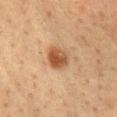Clinical impression:
No biopsy was performed on this lesion — it was imaged during a full skin examination and was not determined to be concerning.
Context:
On the chest. Automated tile analysis of the lesion measured a lesion color around L≈43 a*≈18 b*≈30 in CIELAB and a lesion-to-skin contrast of about 9 (normalized; higher = more distinct). And it measured a border-irregularity rating of about 1.5/10, a color-variation rating of about 4.5/10, and radial color variation of about 1.5. The analysis additionally found a nevus-likeness score of about 95/100 and a detector confidence of about 100 out of 100 that the crop contains a lesion. A female subject, in their mid- to late 50s. A 15 mm close-up extracted from a 3D total-body photography capture.